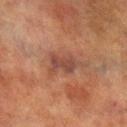follow-up: total-body-photography surveillance lesion; no biopsy
automated lesion analysis: a footprint of about 8 mm², an outline eccentricity of about 0.85 (0 = round, 1 = elongated), and a shape-asymmetry score of about 0.35 (0 = symmetric); about 7 CIELAB-L* units darker than the surrounding skin and a lesion-to-skin contrast of about 7 (normalized; higher = more distinct); a classifier nevus-likeness of about 0/100 and a detector confidence of about 100 out of 100 that the crop contains a lesion
anatomic site: the left lower leg
size: ≈5 mm
acquisition: 15 mm crop, total-body photography
patient: male, aged approximately 70
lighting: cross-polarized illumination